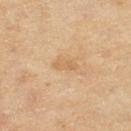biopsy_status: not biopsied; imaged during a skin examination
image:
  source: total-body photography crop
  field_of_view_mm: 15
site: left thigh
patient:
  sex: female
  age_approx: 60